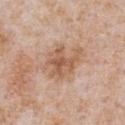Impression:
This lesion was catalogued during total-body skin photography and was not selected for biopsy.
Clinical summary:
On the chest. Cropped from a whole-body photographic skin survey; the tile spans about 15 mm. A male patient aged 63 to 67. The tile uses white-light illumination. The lesion's longest dimension is about 5 mm.